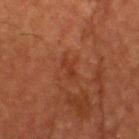The tile uses cross-polarized illumination.
A male patient aged approximately 60.
From the head or neck.
Cropped from a total-body skin-imaging series; the visible field is about 15 mm.